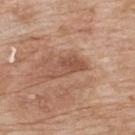Impression: No biopsy was performed on this lesion — it was imaged during a full skin examination and was not determined to be concerning. Context: Located on the upper back. A lesion tile, about 15 mm wide, cut from a 3D total-body photograph. This is a white-light tile. The subject is a female aged approximately 65.